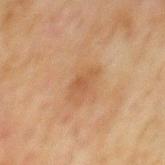This is a cross-polarized tile. A male patient, aged 68–72. Located on the mid back. Measured at roughly 3 mm in maximum diameter. A region of skin cropped from a whole-body photographic capture, roughly 15 mm wide.